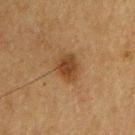follow-up: total-body-photography surveillance lesion; no biopsy
location: the chest
image source: 15 mm crop, total-body photography
patient: male, approximately 75 years of age